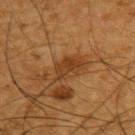Clinical impression: The lesion was tiled from a total-body skin photograph and was not biopsied. Acquisition and patient details: The lesion is on the back. A 15 mm close-up tile from a total-body photography series done for melanoma screening. A male patient, roughly 65 years of age. Approximately 3.5 mm at its widest. The tile uses cross-polarized illumination.